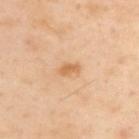<record>
  <biopsy_status>not biopsied; imaged during a skin examination</biopsy_status>
  <image>
    <source>total-body photography crop</source>
    <field_of_view_mm>15</field_of_view_mm>
  </image>
  <site>upper back</site>
  <patient>
    <sex>male</sex>
    <age_approx>55</age_approx>
  </patient>
  <automated_metrics>
    <nevus_likeness_0_100>55</nevus_likeness_0_100>
    <lesion_detection_confidence_0_100>100</lesion_detection_confidence_0_100>
  </automated_metrics>
  <lesion_size>
    <long_diameter_mm_approx>2.5</long_diameter_mm_approx>
  </lesion_size>
</record>